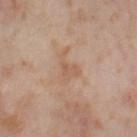Clinical impression:
Captured during whole-body skin photography for melanoma surveillance; the lesion was not biopsied.
Clinical summary:
Automated tile analysis of the lesion measured an area of roughly 5 mm². The analysis additionally found an average lesion color of about L≈58 a*≈18 b*≈31 (CIELAB), about 6 CIELAB-L* units darker than the surrounding skin, and a normalized lesion–skin contrast near 5. And it measured a classifier nevus-likeness of about 0/100 and lesion-presence confidence of about 100/100. This is a cross-polarized tile. A female patient, aged approximately 55. A region of skin cropped from a whole-body photographic capture, roughly 15 mm wide. The lesion is on the right thigh. The recorded lesion diameter is about 3.5 mm.